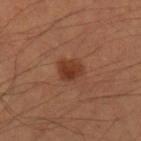follow-up: total-body-photography surveillance lesion; no biopsy | anatomic site: the left upper arm | imaging modality: ~15 mm tile from a whole-body skin photo | subject: male, aged around 35.